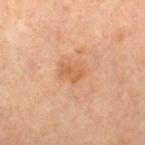Captured during whole-body skin photography for melanoma surveillance; the lesion was not biopsied. A close-up tile cropped from a whole-body skin photograph, about 15 mm across. The subject is a female aged approximately 50. The lesion is on the right thigh.